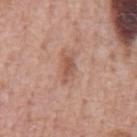Q: Was this lesion biopsied?
A: total-body-photography surveillance lesion; no biopsy
Q: Lesion location?
A: the chest
Q: Patient demographics?
A: male, aged approximately 60
Q: How large is the lesion?
A: ~3 mm (longest diameter)
Q: How was this image acquired?
A: 15 mm crop, total-body photography
Q: Illumination type?
A: white-light illumination